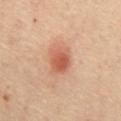The lesion was tiled from a total-body skin photograph and was not biopsied. An algorithmic analysis of the crop reported an average lesion color of about L≈56 a*≈26 b*≈33 (CIELAB) and roughly 11 lightness units darker than nearby skin. This is a cross-polarized tile. Located on the chest. A female patient aged around 65. A close-up tile cropped from a whole-body skin photograph, about 15 mm across.